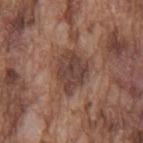| field | value |
|---|---|
| biopsy status | total-body-photography surveillance lesion; no biopsy |
| body site | the mid back |
| lighting | white-light |
| lesion size | about 5 mm |
| automated metrics | an average lesion color of about L≈42 a*≈19 b*≈24 (CIELAB), a lesion–skin lightness drop of about 10, and a lesion-to-skin contrast of about 8 (normalized; higher = more distinct); a classifier nevus-likeness of about 0/100 and a lesion-detection confidence of about 70/100 |
| imaging modality | ~15 mm tile from a whole-body skin photo |
| subject | male, in their mid- to late 70s |